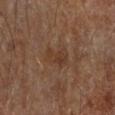<record>
  <biopsy_status>not biopsied; imaged during a skin examination</biopsy_status>
  <lesion_size>
    <long_diameter_mm_approx>3.5</long_diameter_mm_approx>
  </lesion_size>
  <lighting>cross-polarized</lighting>
  <automated_metrics>
    <area_mm2_approx>5.5</area_mm2_approx>
    <eccentricity>0.8</eccentricity>
    <shape_asymmetry>0.45</shape_asymmetry>
    <border_irregularity_0_10>4.5</border_irregularity_0_10>
    <peripheral_color_asymmetry>1.0</peripheral_color_asymmetry>
  </automated_metrics>
  <patient>
    <sex>male</sex>
    <age_approx>85+</age_approx>
  </patient>
  <site>right forearm</site>
  <image>
    <source>total-body photography crop</source>
    <field_of_view_mm>15</field_of_view_mm>
  </image>
</record>